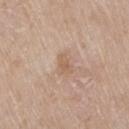Captured during whole-body skin photography for melanoma surveillance; the lesion was not biopsied. This is a white-light tile. A region of skin cropped from a whole-body photographic capture, roughly 15 mm wide. Longest diameter approximately 2.5 mm. A female subject, aged 73 to 77. On the right thigh.